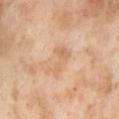Q: Was this lesion biopsied?
A: catalogued during a skin exam; not biopsied
Q: Lesion location?
A: the right thigh
Q: Patient demographics?
A: female, aged 53–57
Q: How was this image acquired?
A: total-body-photography crop, ~15 mm field of view